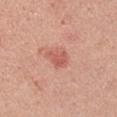The lesion was tiled from a total-body skin photograph and was not biopsied. A male patient, aged approximately 25. A 15 mm close-up tile from a total-body photography series done for melanoma screening. Imaged with white-light lighting. The lesion is on the left upper arm.